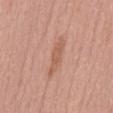Impression:
The lesion was photographed on a routine skin check and not biopsied; there is no pathology result.
Background:
This is a white-light tile. The lesion's longest dimension is about 5 mm. A 15 mm close-up extracted from a 3D total-body photography capture. The lesion is on the abdomen. A female patient, aged 68–72.